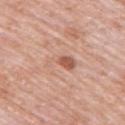Clinical impression: Captured during whole-body skin photography for melanoma surveillance; the lesion was not biopsied. Acquisition and patient details: From the right upper arm. A male subject, roughly 80 years of age. A 15 mm crop from a total-body photograph taken for skin-cancer surveillance.